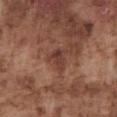follow-up: total-body-photography surveillance lesion; no biopsy
acquisition: ~15 mm tile from a whole-body skin photo
subject: male, approximately 75 years of age
lesion size: about 2.5 mm
anatomic site: the chest
tile lighting: white-light illumination